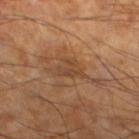Imaged during a routine full-body skin examination; the lesion was not biopsied and no histopathology is available. Imaged with cross-polarized lighting. The lesion is on the left lower leg. The recorded lesion diameter is about 3.5 mm. A male patient aged approximately 60. Cropped from a total-body skin-imaging series; the visible field is about 15 mm. Automated tile analysis of the lesion measured a border-irregularity index near 6/10, internal color variation of about 2.5 on a 0–10 scale, and peripheral color asymmetry of about 0.5. It also reported an automated nevus-likeness rating near 0 out of 100 and a detector confidence of about 70 out of 100 that the crop contains a lesion.